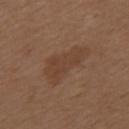Approximately 6.5 mm at its widest. A male patient, in their mid-70s. The lesion is on the mid back. A 15 mm close-up tile from a total-body photography series done for melanoma screening.